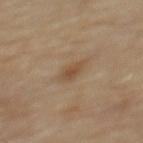{"automated_metrics": {"area_mm2_approx": 3.5, "eccentricity": 0.75, "border_irregularity_0_10": 2.0, "color_variation_0_10": 1.5, "peripheral_color_asymmetry": 0.5, "lesion_detection_confidence_0_100": 100}, "lighting": "cross-polarized", "image": {"source": "total-body photography crop", "field_of_view_mm": 15}, "lesion_size": {"long_diameter_mm_approx": 2.5}, "site": "mid back", "patient": {"sex": "male", "age_approx": "85+"}}